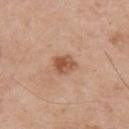Assessment: The lesion was photographed on a routine skin check and not biopsied; there is no pathology result. Background: A region of skin cropped from a whole-body photographic capture, roughly 15 mm wide. The lesion-visualizer software estimated an average lesion color of about L≈54 a*≈23 b*≈33 (CIELAB), about 12 CIELAB-L* units darker than the surrounding skin, and a normalized border contrast of about 8. About 2.5 mm across. On the back. A male patient aged around 55.